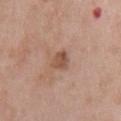{
  "lighting": "white-light",
  "lesion_size": {
    "long_diameter_mm_approx": 2.5
  },
  "image": {
    "source": "total-body photography crop",
    "field_of_view_mm": 15
  },
  "site": "chest",
  "automated_metrics": {
    "area_mm2_approx": 4.5,
    "lesion_detection_confidence_0_100": 100
  },
  "patient": {
    "sex": "male",
    "age_approx": 65
  }
}